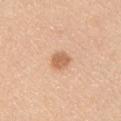Context:
Longest diameter approximately 2.5 mm. A male patient, aged 33 to 37. The total-body-photography lesion software estimated an area of roughly 4.5 mm², a shape eccentricity near 0.6, and a symmetry-axis asymmetry near 0.25. On the chest. Cropped from a total-body skin-imaging series; the visible field is about 15 mm.